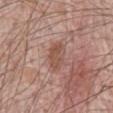Assessment:
Part of a total-body skin-imaging series; this lesion was reviewed on a skin check and was not flagged for biopsy.
Background:
A male patient, approximately 70 years of age. A 15 mm crop from a total-body photograph taken for skin-cancer surveillance. The lesion is located on the front of the torso. Imaged with white-light lighting. About 3.5 mm across. Automated tile analysis of the lesion measured a mean CIELAB color near L≈51 a*≈21 b*≈27. And it measured a nevus-likeness score of about 0/100 and a lesion-detection confidence of about 100/100.